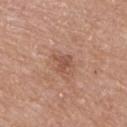The lesion is located on the upper back.
A 15 mm close-up extracted from a 3D total-body photography capture.
A male patient, in their mid-70s.